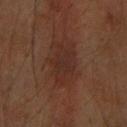| key | value |
|---|---|
| notes | imaged on a skin check; not biopsied |
| body site | the right forearm |
| patient | female, aged 68–72 |
| lesion size | ~4.5 mm (longest diameter) |
| illumination | cross-polarized illumination |
| TBP lesion metrics | an area of roughly 8.5 mm² and two-axis asymmetry of about 0.35; border irregularity of about 3.5 on a 0–10 scale, internal color variation of about 2 on a 0–10 scale, and a peripheral color-asymmetry measure near 0.5; an automated nevus-likeness rating near 0 out of 100 |
| image | total-body-photography crop, ~15 mm field of view |